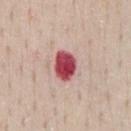Captured during whole-body skin photography for melanoma surveillance; the lesion was not biopsied.
About 3.5 mm across.
The patient is a male roughly 40 years of age.
On the chest.
A close-up tile cropped from a whole-body skin photograph, about 15 mm across.
The lesion-visualizer software estimated a mean CIELAB color near L≈49 a*≈37 b*≈21, about 21 CIELAB-L* units darker than the surrounding skin, and a lesion-to-skin contrast of about 14 (normalized; higher = more distinct).
Imaged with white-light lighting.